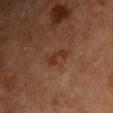Impression: This lesion was catalogued during total-body skin photography and was not selected for biopsy. Context: This is a cross-polarized tile. A male subject, roughly 60 years of age. A region of skin cropped from a whole-body photographic capture, roughly 15 mm wide. The lesion is on the upper back.